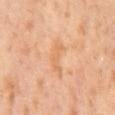No biopsy was performed on this lesion — it was imaged during a full skin examination and was not determined to be concerning. Automated image analysis of the tile measured a color-variation rating of about 1/10. And it measured a nevus-likeness score of about 0/100. The subject is a male in their mid-60s. This image is a 15 mm lesion crop taken from a total-body photograph. The lesion's longest dimension is about 4 mm.